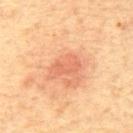The lesion was tiled from a total-body skin photograph and was not biopsied.
Imaged with cross-polarized lighting.
From the mid back.
An algorithmic analysis of the crop reported an area of roughly 6 mm², a shape eccentricity near 0.85, and two-axis asymmetry of about 0.25. The software also gave a mean CIELAB color near L≈59 a*≈27 b*≈34 and a lesion–skin lightness drop of about 8. The software also gave a border-irregularity rating of about 3/10, a within-lesion color-variation index near 1.5/10, and peripheral color asymmetry of about 0.5.
A roughly 15 mm field-of-view crop from a total-body skin photograph.
Approximately 3.5 mm at its widest.
A male patient, aged around 65.